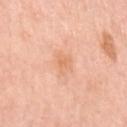<tbp_lesion>
<biopsy_status>not biopsied; imaged during a skin examination</biopsy_status>
<patient>
  <sex>female</sex>
  <age_approx>65</age_approx>
</patient>
<lesion_size>
  <long_diameter_mm_approx>2.5</long_diameter_mm_approx>
</lesion_size>
<site>arm</site>
<image>
  <source>total-body photography crop</source>
  <field_of_view_mm>15</field_of_view_mm>
</image>
</tbp_lesion>